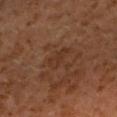Part of a total-body skin-imaging series; this lesion was reviewed on a skin check and was not flagged for biopsy. Measured at roughly 3 mm in maximum diameter. Imaged with cross-polarized lighting. A 15 mm crop from a total-body photograph taken for skin-cancer surveillance. The subject is a male aged approximately 60. On the right forearm.